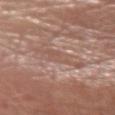workup: no biopsy performed (imaged during a skin exam) | TBP lesion metrics: a footprint of about 6.5 mm² and two-axis asymmetry of about 0.45; an average lesion color of about L≈54 a*≈19 b*≈25 (CIELAB), about 7 CIELAB-L* units darker than the surrounding skin, and a lesion-to-skin contrast of about 5 (normalized; higher = more distinct); a border-irregularity rating of about 6.5/10, a within-lesion color-variation index near 1/10, and peripheral color asymmetry of about 0.5 | subject: male, about 65 years old | illumination: white-light illumination | size: ≈4.5 mm | image: ~15 mm crop, total-body skin-cancer survey | location: the left forearm.